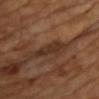Case summary:
- workup: total-body-photography surveillance lesion; no biopsy
- image source: 15 mm crop, total-body photography
- location: the head or neck